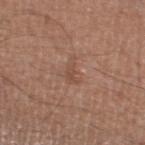Clinical impression: The lesion was tiled from a total-body skin photograph and was not biopsied. Clinical summary: A male patient, aged 63 to 67. The lesion is located on the left lower leg. Longest diameter approximately 3.5 mm. This is a white-light tile. This image is a 15 mm lesion crop taken from a total-body photograph.